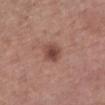Part of a total-body skin-imaging series; this lesion was reviewed on a skin check and was not flagged for biopsy.
A 15 mm crop from a total-body photograph taken for skin-cancer surveillance.
On the left lower leg.
A female patient, in their 50s.
The lesion's longest dimension is about 3 mm.